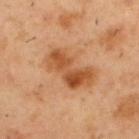From the upper back. Cropped from a total-body skin-imaging series; the visible field is about 15 mm. The patient is a male in their mid-50s. Captured under cross-polarized illumination. The lesion-visualizer software estimated an average lesion color of about L≈52 a*≈24 b*≈39 (CIELAB), a lesion–skin lightness drop of about 11, and a normalized lesion–skin contrast near 8.5. It also reported a border-irregularity rating of about 3.5/10 and radial color variation of about 2.5.